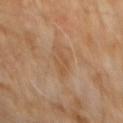The recorded lesion diameter is about 4.5 mm. A male subject, aged approximately 60. On the front of the torso. Captured under cross-polarized illumination. Cropped from a whole-body photographic skin survey; the tile spans about 15 mm.